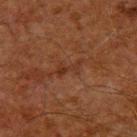Assessment:
This lesion was catalogued during total-body skin photography and was not selected for biopsy.
Image and clinical context:
A male patient aged 58–62. About 3.5 mm across. The lesion is located on the upper back. Cropped from a whole-body photographic skin survey; the tile spans about 15 mm.